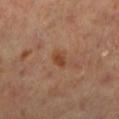<tbp_lesion>
  <biopsy_status>not biopsied; imaged during a skin examination</biopsy_status>
  <image>
    <source>total-body photography crop</source>
    <field_of_view_mm>15</field_of_view_mm>
  </image>
  <lighting>cross-polarized</lighting>
  <automated_metrics>
    <eccentricity>0.75</eccentricity>
    <shape_asymmetry>0.2</shape_asymmetry>
    <border_irregularity_0_10>2.0</border_irregularity_0_10>
    <color_variation_0_10>2.0</color_variation_0_10>
    <peripheral_color_asymmetry>0.5</peripheral_color_asymmetry>
    <nevus_likeness_0_100>20</nevus_likeness_0_100>
    <lesion_detection_confidence_0_100>100</lesion_detection_confidence_0_100>
  </automated_metrics>
  <site>left lower leg</site>
  <lesion_size>
    <long_diameter_mm_approx>2.5</long_diameter_mm_approx>
  </lesion_size>
  <patient>
    <sex>female</sex>
    <age_approx>70</age_approx>
  </patient>
</tbp_lesion>